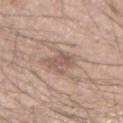workup: catalogued during a skin exam; not biopsied
site: the right forearm
acquisition: 15 mm crop, total-body photography
tile lighting: white-light
diameter: ≈3.5 mm
subject: male, about 45 years old